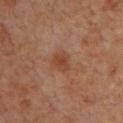Q: Is there a histopathology result?
A: imaged on a skin check; not biopsied
Q: Lesion size?
A: about 2.5 mm
Q: Lesion location?
A: the upper back
Q: Automated lesion metrics?
A: an area of roughly 4.5 mm² and a symmetry-axis asymmetry near 0.3
Q: What kind of image is this?
A: total-body-photography crop, ~15 mm field of view
Q: Who is the patient?
A: male, roughly 60 years of age